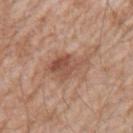workup: total-body-photography surveillance lesion; no biopsy | subject: male, in their 60s | imaging modality: 15 mm crop, total-body photography | anatomic site: the right upper arm.